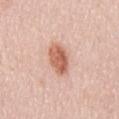biopsy_status: not biopsied; imaged during a skin examination
image:
  source: total-body photography crop
  field_of_view_mm: 15
site: mid back
lighting: white-light
lesion_size:
  long_diameter_mm_approx: 4.5
patient:
  sex: female
  age_approx: 50